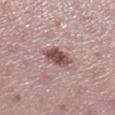site: left lower leg
lesion_size:
  long_diameter_mm_approx: 4.0
lighting: white-light
patient:
  sex: female
  age_approx: 45
image:
  source: total-body photography crop
  field_of_view_mm: 15